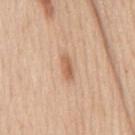| key | value |
|---|---|
| follow-up | total-body-photography surveillance lesion; no biopsy |